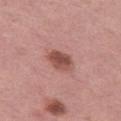| feature | finding |
|---|---|
| anatomic site | the left thigh |
| imaging modality | ~15 mm crop, total-body skin-cancer survey |
| patient | female, aged approximately 50 |
| tile lighting | white-light illumination |